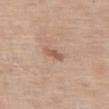No biopsy was performed on this lesion — it was imaged during a full skin examination and was not determined to be concerning. A 15 mm close-up extracted from a 3D total-body photography capture. About 2.5 mm across. A female patient aged 63 to 67. This is a white-light tile. Located on the abdomen.